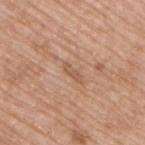Impression:
The lesion was tiled from a total-body skin photograph and was not biopsied.